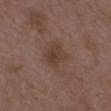The lesion was tiled from a total-body skin photograph and was not biopsied. A roughly 15 mm field-of-view crop from a total-body skin photograph. A female patient, roughly 30 years of age. The lesion is on the arm.